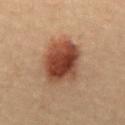Imaged during a routine full-body skin examination; the lesion was not biopsied and no histopathology is available. Imaged with cross-polarized lighting. On the front of the torso. Measured at roughly 5 mm in maximum diameter. The total-body-photography lesion software estimated a lesion area of about 19 mm², an outline eccentricity of about 0.45 (0 = round, 1 = elongated), and two-axis asymmetry of about 0.15. And it measured a border-irregularity index near 1.5/10 and a peripheral color-asymmetry measure near 1.5. The software also gave an automated nevus-likeness rating near 100 out of 100 and a detector confidence of about 100 out of 100 that the crop contains a lesion. Cropped from a whole-body photographic skin survey; the tile spans about 15 mm. A female patient, about 20 years old.